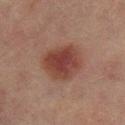Q: Lesion size?
A: ~5 mm (longest diameter)
Q: What kind of image is this?
A: total-body-photography crop, ~15 mm field of view
Q: Lesion location?
A: the right thigh
Q: Patient demographics?
A: female, aged 53 to 57
Q: Illumination type?
A: cross-polarized